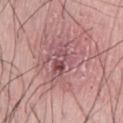Impression:
The lesion was tiled from a total-body skin photograph and was not biopsied.
Context:
A 15 mm close-up tile from a total-body photography series done for melanoma screening. Imaged with white-light lighting. An algorithmic analysis of the crop reported a lesion area of about 8 mm² and a symmetry-axis asymmetry near 0.35. And it measured a lesion color around L≈51 a*≈25 b*≈17 in CIELAB, a lesion–skin lightness drop of about 9, and a normalized border contrast of about 6.5. It also reported border irregularity of about 4 on a 0–10 scale, a color-variation rating of about 8/10, and peripheral color asymmetry of about 3. A male patient, aged approximately 50. The lesion is located on the leg. The recorded lesion diameter is about 4.5 mm.